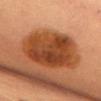Notes:
• follow-up · catalogued during a skin exam; not biopsied
• anatomic site · the chest
• image · ~15 mm crop, total-body skin-cancer survey
• lesion diameter · ≈8 mm
• subject · female, aged approximately 60
• TBP lesion metrics · a footprint of about 36 mm², a shape eccentricity near 0.7, and a symmetry-axis asymmetry near 0.15; a lesion color around L≈39 a*≈24 b*≈34 in CIELAB and a lesion-to-skin contrast of about 10 (normalized; higher = more distinct); a border-irregularity rating of about 2/10; an automated nevus-likeness rating near 100 out of 100 and lesion-presence confidence of about 100/100